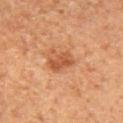{
  "biopsy_status": "not biopsied; imaged during a skin examination",
  "lighting": "cross-polarized",
  "site": "lower back",
  "automated_metrics": {
    "cielab_L": 51,
    "cielab_a": 26,
    "cielab_b": 37,
    "vs_skin_darker_L": 10.0,
    "border_irregularity_0_10": 2.0,
    "peripheral_color_asymmetry": 1.0
  },
  "patient": {
    "sex": "male",
    "age_approx": 65
  },
  "image": {
    "source": "total-body photography crop",
    "field_of_view_mm": 15
  },
  "lesion_size": {
    "long_diameter_mm_approx": 3.0
  }
}